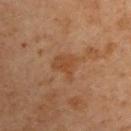Longest diameter approximately 3 mm. Captured under cross-polarized illumination. Automated image analysis of the tile measured an area of roughly 6 mm² and a shape-asymmetry score of about 0.4 (0 = symmetric). And it measured a lesion color around L≈34 a*≈17 b*≈27 in CIELAB and a normalized border contrast of about 5.5. And it measured border irregularity of about 4 on a 0–10 scale, a color-variation rating of about 2/10, and peripheral color asymmetry of about 0.5. A 15 mm close-up extracted from a 3D total-body photography capture. The lesion is located on the right upper arm. A male patient roughly 60 years of age.